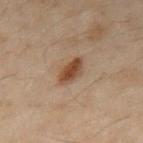The lesion was tiled from a total-body skin photograph and was not biopsied.
The lesion-visualizer software estimated an outline eccentricity of about 0.85 (0 = round, 1 = elongated) and two-axis asymmetry of about 0.2. The analysis additionally found about 10 CIELAB-L* units darker than the surrounding skin and a lesion-to-skin contrast of about 9.5 (normalized; higher = more distinct). It also reported a border-irregularity index near 2.5/10, a within-lesion color-variation index near 3/10, and peripheral color asymmetry of about 1.
From the mid back.
The tile uses cross-polarized illumination.
A roughly 15 mm field-of-view crop from a total-body skin photograph.
The subject is a male aged 48 to 52.
About 3.5 mm across.